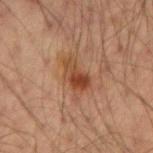Located on the arm.
The total-body-photography lesion software estimated an average lesion color of about L≈42 a*≈22 b*≈31 (CIELAB) and a normalized lesion–skin contrast near 8.5. It also reported border irregularity of about 4 on a 0–10 scale, a within-lesion color-variation index near 7/10, and radial color variation of about 2.5. And it measured an automated nevus-likeness rating near 45 out of 100 and lesion-presence confidence of about 100/100.
This image is a 15 mm lesion crop taken from a total-body photograph.
A male patient aged 33–37.
The tile uses cross-polarized illumination.